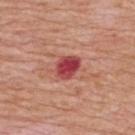This lesion was catalogued during total-body skin photography and was not selected for biopsy. A male patient aged approximately 65. The lesion is on the back. The lesion-visualizer software estimated a lesion area of about 6.5 mm², an eccentricity of roughly 0.65, and a symmetry-axis asymmetry near 0.25. The software also gave a border-irregularity rating of about 2/10, a within-lesion color-variation index near 4/10, and peripheral color asymmetry of about 1.5. And it measured lesion-presence confidence of about 100/100. A lesion tile, about 15 mm wide, cut from a 3D total-body photograph.